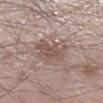{"biopsy_status": "not biopsied; imaged during a skin examination", "lighting": "white-light", "patient": {"sex": "male", "age_approx": 35}, "site": "right lower leg", "image": {"source": "total-body photography crop", "field_of_view_mm": 15}}